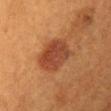Located on the chest.
A female patient in their 40s.
A roughly 15 mm field-of-view crop from a total-body skin photograph.
The lesion-visualizer software estimated border irregularity of about 2 on a 0–10 scale, internal color variation of about 3.5 on a 0–10 scale, and a peripheral color-asymmetry measure near 1. It also reported a classifier nevus-likeness of about 95/100.